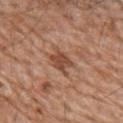The lesion was photographed on a routine skin check and not biopsied; there is no pathology result. Measured at roughly 3.5 mm in maximum diameter. The lesion is located on the upper back. The patient is a male aged 78–82. Cropped from a whole-body photographic skin survey; the tile spans about 15 mm.